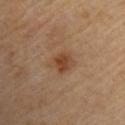Recorded during total-body skin imaging; not selected for excision or biopsy. The tile uses cross-polarized illumination. Measured at roughly 3 mm in maximum diameter. A 15 mm crop from a total-body photograph taken for skin-cancer surveillance. On the right upper arm. Automated tile analysis of the lesion measured a lesion color around L≈43 a*≈19 b*≈31 in CIELAB and a normalized border contrast of about 7.5. The software also gave a nevus-likeness score of about 80/100 and lesion-presence confidence of about 100/100. A male subject aged 83 or over.